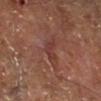Findings:
– workup — catalogued during a skin exam; not biopsied
– size — ≈2.5 mm
– site — the right lower leg
– acquisition — ~15 mm tile from a whole-body skin photo
– patient — in their mid-60s